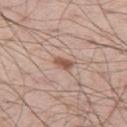biopsy status=catalogued during a skin exam; not biopsied
automated lesion analysis=a lesion–skin lightness drop of about 12 and a normalized lesion–skin contrast near 8
tile lighting=white-light illumination
patient=male, approximately 50 years of age
size=≈2.5 mm
image source=15 mm crop, total-body photography
anatomic site=the left thigh